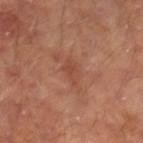biopsy status = no biopsy performed (imaged during a skin exam)
image source = ~15 mm crop, total-body skin-cancer survey
diameter = about 3.5 mm
body site = the leg
subject = male, aged approximately 65
illumination = cross-polarized
TBP lesion metrics = a lesion color around L≈45 a*≈24 b*≈30 in CIELAB, a lesion–skin lightness drop of about 6, and a normalized border contrast of about 5; a border-irregularity index near 3.5/10, internal color variation of about 2 on a 0–10 scale, and peripheral color asymmetry of about 1; a detector confidence of about 100 out of 100 that the crop contains a lesion